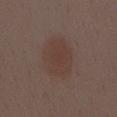Recorded during total-body skin imaging; not selected for excision or biopsy.
The recorded lesion diameter is about 5.5 mm.
A close-up tile cropped from a whole-body skin photograph, about 15 mm across.
Imaged with white-light lighting.
The lesion-visualizer software estimated border irregularity of about 1.5 on a 0–10 scale, internal color variation of about 2 on a 0–10 scale, and a peripheral color-asymmetry measure near 1.
A female patient, in their 50s.
On the abdomen.